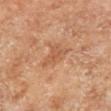Clinical impression: This lesion was catalogued during total-body skin photography and was not selected for biopsy. Image and clinical context: The total-body-photography lesion software estimated a footprint of about 7 mm², an eccentricity of roughly 0.7, and two-axis asymmetry of about 0.45. It also reported a border-irregularity rating of about 4/10 and peripheral color asymmetry of about 1. It also reported a nevus-likeness score of about 0/100 and a detector confidence of about 100 out of 100 that the crop contains a lesion. The subject is a male roughly 70 years of age. The lesion is located on the right lower leg. A close-up tile cropped from a whole-body skin photograph, about 15 mm across. The recorded lesion diameter is about 3.5 mm.